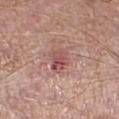Case summary:
- notes — imaged on a skin check; not biopsied
- patient — male, approximately 55 years of age
- lesion size — about 3 mm
- site — the left lower leg
- imaging modality — 15 mm crop, total-body photography
- TBP lesion metrics — an automated nevus-likeness rating near 0 out of 100
- illumination — white-light